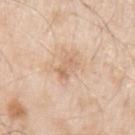{"lighting": "white-light", "lesion_size": {"long_diameter_mm_approx": 3.5}, "patient": {"sex": "male", "age_approx": 55}, "site": "left upper arm", "image": {"source": "total-body photography crop", "field_of_view_mm": 15}}